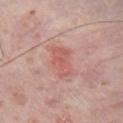Clinical impression: Captured during whole-body skin photography for melanoma surveillance; the lesion was not biopsied. Background: The lesion is located on the chest. The lesion's longest dimension is about 4 mm. A lesion tile, about 15 mm wide, cut from a 3D total-body photograph. The subject is a male aged around 55. Automated image analysis of the tile measured a footprint of about 8 mm², a shape eccentricity near 0.8, and a symmetry-axis asymmetry near 0.3. The analysis additionally found a lesion–skin lightness drop of about 7. It also reported a border-irregularity index near 3/10, a within-lesion color-variation index near 2.5/10, and a peripheral color-asymmetry measure near 0.5. The software also gave a classifier nevus-likeness of about 55/100. This is a white-light tile.